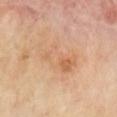Assessment:
The lesion was photographed on a routine skin check and not biopsied; there is no pathology result.
Context:
The subject is a male aged 68 to 72. Imaged with cross-polarized lighting. A roughly 15 mm field-of-view crop from a total-body skin photograph. The recorded lesion diameter is about 6.5 mm. From the chest.